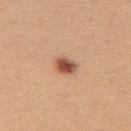biopsy_status: not biopsied; imaged during a skin examination
patient:
  sex: female
  age_approx: 25
image:
  source: total-body photography crop
  field_of_view_mm: 15
site: upper back